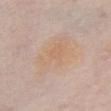notes: imaged on a skin check; not biopsied | tile lighting: white-light | lesion diameter: ≈8 mm | site: the front of the torso | TBP lesion metrics: a border-irregularity rating of about 3.5/10, internal color variation of about 3.5 on a 0–10 scale, and a peripheral color-asymmetry measure near 1 | imaging modality: total-body-photography crop, ~15 mm field of view | subject: female, roughly 65 years of age.